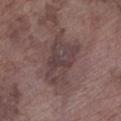A male subject, roughly 75 years of age. The lesion is on the left lower leg. An algorithmic analysis of the crop reported a border-irregularity rating of about 4/10, a color-variation rating of about 4/10, and peripheral color asymmetry of about 1.5. The lesion's longest dimension is about 6.5 mm. Captured under white-light illumination. A 15 mm close-up extracted from a 3D total-body photography capture.